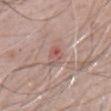On the front of the torso.
The lesion's longest dimension is about 2.5 mm.
Imaged with white-light lighting.
The subject is a male in their 70s.
A roughly 15 mm field-of-view crop from a total-body skin photograph.
Automated tile analysis of the lesion measured border irregularity of about 3 on a 0–10 scale, a color-variation rating of about 6/10, and a peripheral color-asymmetry measure near 1.5.